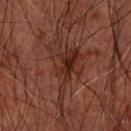Assessment: The lesion was photographed on a routine skin check and not biopsied; there is no pathology result. Image and clinical context: The lesion-visualizer software estimated a lesion color around L≈23 a*≈18 b*≈21 in CIELAB, about 6 CIELAB-L* units darker than the surrounding skin, and a normalized border contrast of about 7.5. It also reported a border-irregularity rating of about 10/10, a color-variation rating of about 6.5/10, and a peripheral color-asymmetry measure near 2.5. A male patient in their 70s. On the arm. A 15 mm close-up tile from a total-body photography series done for melanoma screening. Imaged with cross-polarized lighting.